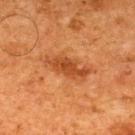• workup · catalogued during a skin exam; not biopsied
• body site · the back
• image · ~15 mm tile from a whole-body skin photo
• image-analysis metrics · a lesion area of about 10 mm² and a shape-asymmetry score of about 0.3 (0 = symmetric); a border-irregularity rating of about 4/10 and internal color variation of about 3 on a 0–10 scale
• patient · male, aged around 65
• lesion size · ≈5.5 mm